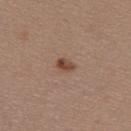Impression: Recorded during total-body skin imaging; not selected for excision or biopsy. Acquisition and patient details: Located on the back. A region of skin cropped from a whole-body photographic capture, roughly 15 mm wide. A female subject, aged 28 to 32.